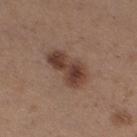follow-up=no biopsy performed (imaged during a skin exam)
tile lighting=white-light illumination
lesion diameter=≈5.5 mm
image source=~15 mm crop, total-body skin-cancer survey
image-analysis metrics=an area of roughly 11 mm² and an eccentricity of roughly 0.9; a mean CIELAB color near L≈40 a*≈19 b*≈25, about 12 CIELAB-L* units darker than the surrounding skin, and a normalized border contrast of about 9.5; a border-irregularity index near 3.5/10, internal color variation of about 5 on a 0–10 scale, and radial color variation of about 1.5
subject=female, roughly 30 years of age
site=the right thigh